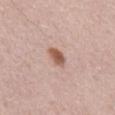Findings:
- notes: no biopsy performed (imaged during a skin exam)
- subject: male, approximately 65 years of age
- location: the mid back
- diameter: ~2.5 mm (longest diameter)
- illumination: white-light illumination
- acquisition: ~15 mm crop, total-body skin-cancer survey
- automated lesion analysis: a footprint of about 4 mm², a shape eccentricity near 0.65, and a symmetry-axis asymmetry near 0.2; an average lesion color of about L≈56 a*≈22 b*≈28 (CIELAB), roughly 13 lightness units darker than nearby skin, and a normalized lesion–skin contrast near 9